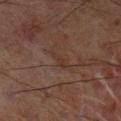<record>
<biopsy_status>not biopsied; imaged during a skin examination</biopsy_status>
<image>
  <source>total-body photography crop</source>
  <field_of_view_mm>15</field_of_view_mm>
</image>
<automated_metrics>
  <border_irregularity_0_10>4.5</border_irregularity_0_10>
  <nevus_likeness_0_100>0</nevus_likeness_0_100>
</automated_metrics>
<site>left lower leg</site>
<patient>
  <sex>male</sex>
  <age_approx>70</age_approx>
</patient>
</record>